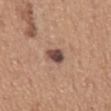| feature | finding |
|---|---|
| biopsy status | catalogued during a skin exam; not biopsied |
| acquisition | ~15 mm crop, total-body skin-cancer survey |
| tile lighting | white-light |
| subject | male, aged 63–67 |
| lesion size | ≈3 mm |
| site | the back |